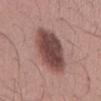workup: no biopsy performed (imaged during a skin exam) | imaging modality: ~15 mm crop, total-body skin-cancer survey | patient: male, aged 33–37 | site: the back | illumination: white-light | automated lesion analysis: border irregularity of about 2 on a 0–10 scale and radial color variation of about 1.5.